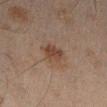The lesion was tiled from a total-body skin photograph and was not biopsied. A roughly 15 mm field-of-view crop from a total-body skin photograph. On the leg. A male subject, approximately 45 years of age. Captured under cross-polarized illumination. The recorded lesion diameter is about 3 mm.